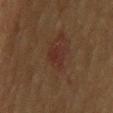Imaged during a routine full-body skin examination; the lesion was not biopsied and no histopathology is available. A 15 mm close-up extracted from a 3D total-body photography capture. The lesion is on the upper back. The subject is a male roughly 85 years of age or older.